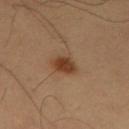Assessment: The lesion was photographed on a routine skin check and not biopsied; there is no pathology result. Background: Located on the mid back. This is a cross-polarized tile. The subject is a male aged approximately 55. Measured at roughly 3 mm in maximum diameter. A close-up tile cropped from a whole-body skin photograph, about 15 mm across.